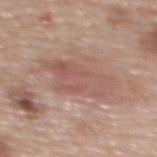The lesion was photographed on a routine skin check and not biopsied; there is no pathology result. The lesion is on the upper back. A 15 mm crop from a total-body photograph taken for skin-cancer surveillance. A female patient about 60 years old.